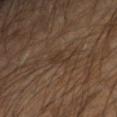Assessment:
Part of a total-body skin-imaging series; this lesion was reviewed on a skin check and was not flagged for biopsy.
Acquisition and patient details:
A male patient, aged around 55. A close-up tile cropped from a whole-body skin photograph, about 15 mm across. Located on the right forearm. An algorithmic analysis of the crop reported a lesion area of about 3.5 mm², a shape eccentricity near 0.85, and a shape-asymmetry score of about 0.5 (0 = symmetric). The analysis additionally found border irregularity of about 5 on a 0–10 scale. Imaged with cross-polarized lighting.